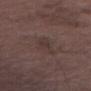Clinical impression:
No biopsy was performed on this lesion — it was imaged during a full skin examination and was not determined to be concerning.
Acquisition and patient details:
The total-body-photography lesion software estimated a border-irregularity rating of about 3.5/10, a color-variation rating of about 1.5/10, and a peripheral color-asymmetry measure near 0.5. The software also gave a detector confidence of about 80 out of 100 that the crop contains a lesion. About 4 mm across. A region of skin cropped from a whole-body photographic capture, roughly 15 mm wide. The patient is a male approximately 75 years of age. Located on the left thigh.